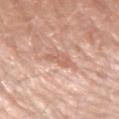workup: total-body-photography surveillance lesion; no biopsy | diameter: ≈2.5 mm | anatomic site: the right upper arm | lighting: white-light | subject: male, aged 78 to 82 | image: ~15 mm tile from a whole-body skin photo | automated metrics: a footprint of about 4 mm², an eccentricity of roughly 0.75, and a shape-asymmetry score of about 0.4 (0 = symmetric); a border-irregularity index near 4/10, a within-lesion color-variation index near 1.5/10, and peripheral color asymmetry of about 0.5; a classifier nevus-likeness of about 0/100 and a detector confidence of about 100 out of 100 that the crop contains a lesion.